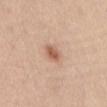| field | value |
|---|---|
| site | the abdomen |
| lesion diameter | ≈3 mm |
| tile lighting | white-light illumination |
| image source | ~15 mm tile from a whole-body skin photo |
| subject | male, in their mid- to late 40s |
| automated metrics | a lesion color around L≈59 a*≈21 b*≈31 in CIELAB, a lesion–skin lightness drop of about 11, and a normalized border contrast of about 7.5 |